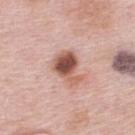No biopsy was performed on this lesion — it was imaged during a full skin examination and was not determined to be concerning.
This image is a 15 mm lesion crop taken from a total-body photograph.
The patient is a female aged approximately 65.
The lesion is located on the back.